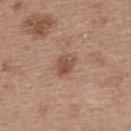No biopsy was performed on this lesion — it was imaged during a full skin examination and was not determined to be concerning. A 15 mm close-up extracted from a 3D total-body photography capture. A female patient, aged around 40. The tile uses white-light illumination. Automated tile analysis of the lesion measured a lesion area of about 4.5 mm², a shape eccentricity near 0.7, and two-axis asymmetry of about 0.2. And it measured roughly 10 lightness units darker than nearby skin. The analysis additionally found a border-irregularity rating of about 2/10, a color-variation rating of about 3/10, and peripheral color asymmetry of about 1. From the upper back.